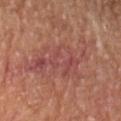Clinical impression:
No biopsy was performed on this lesion — it was imaged during a full skin examination and was not determined to be concerning.
Context:
Imaged with cross-polarized lighting. The total-body-photography lesion software estimated a border-irregularity rating of about 7/10, a within-lesion color-variation index near 5/10, and peripheral color asymmetry of about 1.5. Approximately 10 mm at its widest. The subject is a female aged 68–72. A 15 mm close-up extracted from a 3D total-body photography capture. From the left forearm.